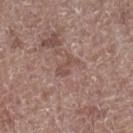workup=total-body-photography surveillance lesion; no biopsy
image source=total-body-photography crop, ~15 mm field of view
patient=male, aged 68 to 72
site=the right lower leg
TBP lesion metrics=a lesion color around L≈49 a*≈19 b*≈23 in CIELAB, about 7 CIELAB-L* units darker than the surrounding skin, and a normalized lesion–skin contrast near 5; a border-irregularity rating of about 6.5/10, a color-variation rating of about 0/10, and a peripheral color-asymmetry measure near 0; an automated nevus-likeness rating near 0 out of 100 and a lesion-detection confidence of about 80/100
lesion size=about 3 mm